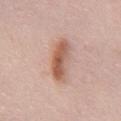The lesion was photographed on a routine skin check and not biopsied; there is no pathology result.
A 15 mm close-up extracted from a 3D total-body photography capture.
On the chest.
A female subject aged approximately 50.
Imaged with white-light lighting.